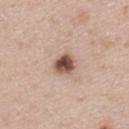Background: A roughly 15 mm field-of-view crop from a total-body skin photograph. Automated image analysis of the tile measured an area of roughly 6 mm², an outline eccentricity of about 0.35 (0 = round, 1 = elongated), and a symmetry-axis asymmetry near 0.15. The software also gave a border-irregularity index near 1.5/10, internal color variation of about 6.5 on a 0–10 scale, and radial color variation of about 2. The lesion is on the upper back. A male subject, approximately 40 years of age. About 3 mm across. The tile uses white-light illumination.